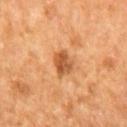Assessment: This lesion was catalogued during total-body skin photography and was not selected for biopsy. Background: A roughly 15 mm field-of-view crop from a total-body skin photograph. From the mid back. Measured at roughly 4 mm in maximum diameter. A male patient roughly 65 years of age.